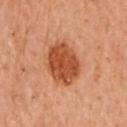Clinical impression:
The lesion was tiled from a total-body skin photograph and was not biopsied.
Context:
The lesion's longest dimension is about 5 mm. Cropped from a whole-body photographic skin survey; the tile spans about 15 mm. A female subject, aged 58 to 62. Imaged with cross-polarized lighting. An algorithmic analysis of the crop reported two-axis asymmetry of about 0.15. The analysis additionally found a border-irregularity rating of about 1.5/10, a within-lesion color-variation index near 3.5/10, and a peripheral color-asymmetry measure near 1. The analysis additionally found an automated nevus-likeness rating near 95 out of 100. The lesion is on the left arm.